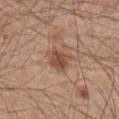Impression: Captured during whole-body skin photography for melanoma surveillance; the lesion was not biopsied. Acquisition and patient details: Approximately 3.5 mm at its widest. A male patient, aged 43–47. A lesion tile, about 15 mm wide, cut from a 3D total-body photograph. The lesion is located on the right upper arm. The lesion-visualizer software estimated a border-irregularity rating of about 2.5/10 and a color-variation rating of about 3/10.